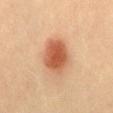An algorithmic analysis of the crop reported a border-irregularity rating of about 1.5/10 and radial color variation of about 1. It also reported a nevus-likeness score of about 100/100 and a detector confidence of about 100 out of 100 that the crop contains a lesion. A male subject aged 38–42. This is a cross-polarized tile. On the mid back. Cropped from a total-body skin-imaging series; the visible field is about 15 mm. About 5 mm across.